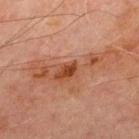<case>
<biopsy_status>not biopsied; imaged during a skin examination</biopsy_status>
<image>
  <source>total-body photography crop</source>
  <field_of_view_mm>15</field_of_view_mm>
</image>
<lighting>cross-polarized</lighting>
<site>chest</site>
<patient>
  <sex>male</sex>
  <age_approx>70</age_approx>
</patient>
<lesion_size>
  <long_diameter_mm_approx>10.0</long_diameter_mm_approx>
</lesion_size>
</case>